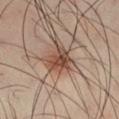Captured during whole-body skin photography for melanoma surveillance; the lesion was not biopsied. The tile uses cross-polarized illumination. The lesion is located on the right thigh. A male patient about 40 years old. A region of skin cropped from a whole-body photographic capture, roughly 15 mm wide. Longest diameter approximately 3.5 mm. Automated image analysis of the tile measured a lesion color around L≈45 a*≈20 b*≈27 in CIELAB and about 12 CIELAB-L* units darker than the surrounding skin. The software also gave border irregularity of about 3 on a 0–10 scale, a color-variation rating of about 6/10, and peripheral color asymmetry of about 2. The analysis additionally found a nevus-likeness score of about 80/100 and a lesion-detection confidence of about 100/100.